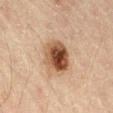follow-up = catalogued during a skin exam; not biopsied | subject = male, aged 43 to 47 | imaging modality = ~15 mm tile from a whole-body skin photo | body site = the abdomen.